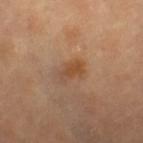Part of a total-body skin-imaging series; this lesion was reviewed on a skin check and was not flagged for biopsy. The patient is a female aged 68–72. The lesion is on the right lower leg. This image is a 15 mm lesion crop taken from a total-body photograph. The tile uses cross-polarized illumination.